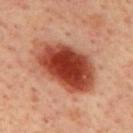Findings:
• workup — imaged on a skin check; not biopsied
• site — the mid back
• subject — male, aged around 65
• lesion diameter — ~8 mm (longest diameter)
• image — ~15 mm tile from a whole-body skin photo
• illumination — cross-polarized
• image-analysis metrics — border irregularity of about 2 on a 0–10 scale, a within-lesion color-variation index near 7.5/10, and radial color variation of about 2; a nevus-likeness score of about 100/100 and a lesion-detection confidence of about 100/100